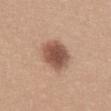Part of a total-body skin-imaging series; this lesion was reviewed on a skin check and was not flagged for biopsy.
On the mid back.
A female subject, roughly 30 years of age.
Longest diameter approximately 4.5 mm.
The total-body-photography lesion software estimated a normalized border contrast of about 10. It also reported a detector confidence of about 100 out of 100 that the crop contains a lesion.
Cropped from a whole-body photographic skin survey; the tile spans about 15 mm.